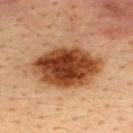Imaged during a routine full-body skin examination; the lesion was not biopsied and no histopathology is available.
Located on the upper back.
The subject is a male aged approximately 35.
The recorded lesion diameter is about 8.5 mm.
A roughly 15 mm field-of-view crop from a total-body skin photograph.
The tile uses cross-polarized illumination.